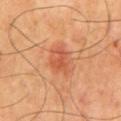Findings:
• notes: total-body-photography surveillance lesion; no biopsy
• acquisition: 15 mm crop, total-body photography
• site: the mid back
• subject: male, about 65 years old